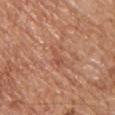follow-up: catalogued during a skin exam; not biopsied
lesion size: ~2.5 mm (longest diameter)
subject: male, roughly 65 years of age
imaging modality: total-body-photography crop, ~15 mm field of view
tile lighting: white-light
anatomic site: the chest